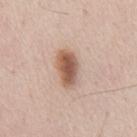The patient is a male roughly 45 years of age. Approximately 4.5 mm at its widest. A 15 mm close-up tile from a total-body photography series done for melanoma screening. Located on the front of the torso.